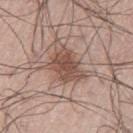Findings:
- workup · no biopsy performed (imaged during a skin exam)